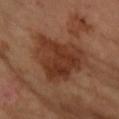The lesion was tiled from a total-body skin photograph and was not biopsied. Captured under cross-polarized illumination. Approximately 7.5 mm at its widest. This image is a 15 mm lesion crop taken from a total-body photograph. A female patient, aged 58–62. The lesion is located on the right forearm.